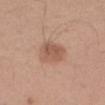Recorded during total-body skin imaging; not selected for excision or biopsy. Measured at roughly 3.5 mm in maximum diameter. Located on the right forearm. This image is a 15 mm lesion crop taken from a total-body photograph. A female patient roughly 40 years of age. Captured under white-light illumination.